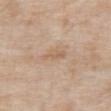Context: Cropped from a total-body skin-imaging series; the visible field is about 15 mm. Located on the abdomen. The subject is a male aged 78–82.